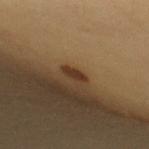workup = no biopsy performed (imaged during a skin exam) | patient = female, in their mid-30s | automated lesion analysis = an area of roughly 2.5 mm², a shape eccentricity near 0.95, and two-axis asymmetry of about 0.35; a mean CIELAB color near L≈34 a*≈18 b*≈29, about 11 CIELAB-L* units darker than the surrounding skin, and a normalized border contrast of about 10; border irregularity of about 3.5 on a 0–10 scale, a color-variation rating of about 0/10, and peripheral color asymmetry of about 0 | imaging modality = ~15 mm tile from a whole-body skin photo | anatomic site = the upper back | diameter = ~3 mm (longest diameter).